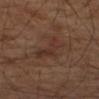| key | value |
|---|---|
| biopsy status | catalogued during a skin exam; not biopsied |
| location | the left forearm |
| subject | male, in their mid-60s |
| illumination | cross-polarized |
| imaging modality | total-body-photography crop, ~15 mm field of view |
| size | ≈5 mm |